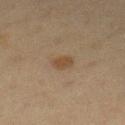Q: Was this lesion biopsied?
A: no biopsy performed (imaged during a skin exam)
Q: What are the patient's age and sex?
A: female, roughly 50 years of age
Q: What is the anatomic site?
A: the left lower leg
Q: How was this image acquired?
A: total-body-photography crop, ~15 mm field of view
Q: Illumination type?
A: cross-polarized
Q: How large is the lesion?
A: ≈2.5 mm